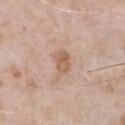Impression: The lesion was tiled from a total-body skin photograph and was not biopsied. Image and clinical context: Longest diameter approximately 3 mm. A region of skin cropped from a whole-body photographic capture, roughly 15 mm wide. Automated tile analysis of the lesion measured two-axis asymmetry of about 0.2. And it measured a lesion–skin lightness drop of about 9 and a normalized lesion–skin contrast near 6.5. Captured under white-light illumination. The lesion is on the chest. The subject is a male aged around 50.